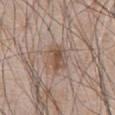Findings:
- biopsy status · total-body-photography surveillance lesion; no biopsy
- subject · male, in their mid-60s
- imaging modality · ~15 mm crop, total-body skin-cancer survey
- TBP lesion metrics · a mean CIELAB color near L≈51 a*≈16 b*≈26, a lesion–skin lightness drop of about 9, and a lesion-to-skin contrast of about 7 (normalized; higher = more distinct)
- diameter · about 3.5 mm
- site · the abdomen
- lighting · white-light